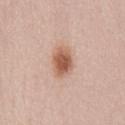follow-up: total-body-photography surveillance lesion; no biopsy | subject: female, roughly 55 years of age | illumination: white-light | site: the mid back | imaging modality: 15 mm crop, total-body photography | TBP lesion metrics: a shape eccentricity near 0.7 and a shape-asymmetry score of about 0.25 (0 = symmetric); a mean CIELAB color near L≈58 a*≈22 b*≈30 and a normalized border contrast of about 9.5; a detector confidence of about 100 out of 100 that the crop contains a lesion.